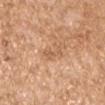Clinical impression:
This lesion was catalogued during total-body skin photography and was not selected for biopsy.
Clinical summary:
Cropped from a whole-body photographic skin survey; the tile spans about 15 mm. Located on the right upper arm. The patient is a male aged 73 to 77.